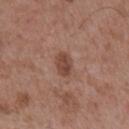Q: Was a biopsy performed?
A: imaged on a skin check; not biopsied
Q: Lesion location?
A: the mid back
Q: Who is the patient?
A: male, roughly 55 years of age
Q: What is the imaging modality?
A: total-body-photography crop, ~15 mm field of view
Q: How large is the lesion?
A: ≈3 mm